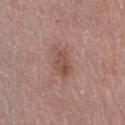{"biopsy_status": "not biopsied; imaged during a skin examination", "image": {"source": "total-body photography crop", "field_of_view_mm": 15}, "lighting": "white-light", "site": "left thigh", "patient": {"sex": "female", "age_approx": 60}}